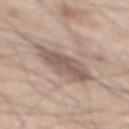Clinical impression: Part of a total-body skin-imaging series; this lesion was reviewed on a skin check and was not flagged for biopsy. Image and clinical context: A male patient, aged around 65. From the mid back. Longest diameter approximately 6 mm. The tile uses white-light illumination. A roughly 15 mm field-of-view crop from a total-body skin photograph. The lesion-visualizer software estimated a lesion area of about 13 mm² and a symmetry-axis asymmetry near 0.2. The analysis additionally found a nevus-likeness score of about 55/100 and lesion-presence confidence of about 85/100.